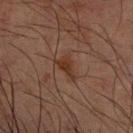This lesion was catalogued during total-body skin photography and was not selected for biopsy.
From the right forearm.
A male patient, in their 70s.
A 15 mm crop from a total-body photograph taken for skin-cancer surveillance.
This is a cross-polarized tile.
Approximately 3 mm at its widest.
Automated image analysis of the tile measured a footprint of about 3.5 mm² and a shape-asymmetry score of about 0.5 (0 = symmetric). The software also gave a lesion color around L≈26 a*≈17 b*≈23 in CIELAB and a normalized lesion–skin contrast near 8.5. It also reported border irregularity of about 5 on a 0–10 scale, a color-variation rating of about 2/10, and radial color variation of about 1.